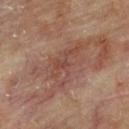No biopsy was performed on this lesion — it was imaged during a full skin examination and was not determined to be concerning. The subject is a male aged approximately 85 or older. A close-up tile cropped from a whole-body skin photograph, about 15 mm across. Located on the upper back. The tile uses cross-polarized illumination.